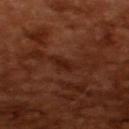Case summary:
– notes: total-body-photography surveillance lesion; no biopsy
– subject: male, aged approximately 65
– lighting: cross-polarized
– image: 15 mm crop, total-body photography